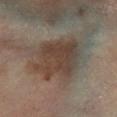workup: no biopsy performed (imaged during a skin exam)
patient: female, aged 63 to 67
lighting: cross-polarized illumination
image: 15 mm crop, total-body photography
TBP lesion metrics: a lesion color around L≈40 a*≈12 b*≈22 in CIELAB, a lesion–skin lightness drop of about 9, and a lesion-to-skin contrast of about 8 (normalized; higher = more distinct); border irregularity of about 3 on a 0–10 scale, a within-lesion color-variation index near 5.5/10, and peripheral color asymmetry of about 1.5; a nevus-likeness score of about 15/100
body site: the left leg
lesion size: ≈6.5 mm